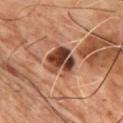follow-up=no biopsy performed (imaged during a skin exam) | patient=male, in their mid-60s | lesion size=about 4 mm | location=the chest | acquisition=~15 mm tile from a whole-body skin photo | tile lighting=cross-polarized illumination.